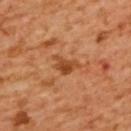The lesion was photographed on a routine skin check and not biopsied; there is no pathology result. The tile uses cross-polarized illumination. Measured at roughly 3.5 mm in maximum diameter. A female subject, aged 53 to 57. From the upper back. A region of skin cropped from a whole-body photographic capture, roughly 15 mm wide.